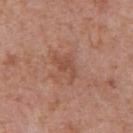Q: Is there a histopathology result?
A: imaged on a skin check; not biopsied
Q: What is the lesion's diameter?
A: ≈3 mm
Q: Automated lesion metrics?
A: a shape eccentricity near 0.8 and two-axis asymmetry of about 0.45; border irregularity of about 5.5 on a 0–10 scale, a within-lesion color-variation index near 0.5/10, and peripheral color asymmetry of about 0; a detector confidence of about 100 out of 100 that the crop contains a lesion
Q: How was this image acquired?
A: total-body-photography crop, ~15 mm field of view
Q: Lesion location?
A: the chest
Q: Patient demographics?
A: male, aged around 70
Q: What lighting was used for the tile?
A: white-light